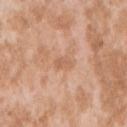Image and clinical context: The patient is a female aged 23 to 27. The lesion's longest dimension is about 2.5 mm. This image is a 15 mm lesion crop taken from a total-body photograph. The tile uses white-light illumination. The lesion is on the right upper arm.